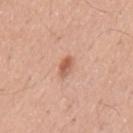Assessment: This lesion was catalogued during total-body skin photography and was not selected for biopsy. Acquisition and patient details: This is a white-light tile. A region of skin cropped from a whole-body photographic capture, roughly 15 mm wide. Located on the mid back. Measured at roughly 3 mm in maximum diameter. A male subject in their mid-50s. Automated tile analysis of the lesion measured an area of roughly 3.5 mm², an eccentricity of roughly 0.85, and a symmetry-axis asymmetry near 0.25.